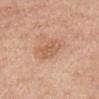No biopsy was performed on this lesion — it was imaged during a full skin examination and was not determined to be concerning. A male subject about 55 years old. A 15 mm close-up extracted from a 3D total-body photography capture. The lesion is located on the left upper arm.